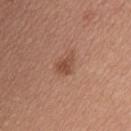biopsy_status: not biopsied; imaged during a skin examination
lesion_size:
  long_diameter_mm_approx: 2.5
image:
  source: total-body photography crop
  field_of_view_mm: 15
patient:
  sex: female
  age_approx: 45
site: upper back
automated_metrics:
  border_irregularity_0_10: 3.5
  color_variation_0_10: 2.0
  peripheral_color_asymmetry: 1.0
  nevus_likeness_0_100: 80
  lesion_detection_confidence_0_100: 100
lighting: white-light